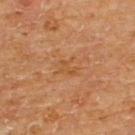Imaged during a routine full-body skin examination; the lesion was not biopsied and no histopathology is available.